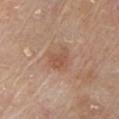{"biopsy_status": "not biopsied; imaged during a skin examination", "patient": {"sex": "male", "age_approx": 80}, "automated_metrics": {"border_irregularity_0_10": 3.0, "color_variation_0_10": 2.5}, "image": {"source": "total-body photography crop", "field_of_view_mm": 15}, "lesion_size": {"long_diameter_mm_approx": 3.0}, "lighting": "white-light", "site": "chest"}